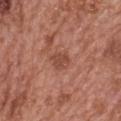Impression: The lesion was photographed on a routine skin check and not biopsied; there is no pathology result. Context: A female patient, aged approximately 60. This image is a 15 mm lesion crop taken from a total-body photograph. Located on the upper back. Captured under white-light illumination.